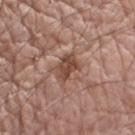The lesion was photographed on a routine skin check and not biopsied; there is no pathology result. A male subject approximately 65 years of age. This is a white-light tile. Automated tile analysis of the lesion measured a lesion color around L≈46 a*≈20 b*≈27 in CIELAB, about 11 CIELAB-L* units darker than the surrounding skin, and a lesion-to-skin contrast of about 8.5 (normalized; higher = more distinct). It also reported a border-irregularity index near 3/10. The software also gave a nevus-likeness score of about 0/100. Cropped from a whole-body photographic skin survey; the tile spans about 15 mm. The lesion is located on the right upper arm.